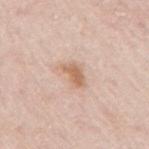Case summary:
- follow-up: no biopsy performed (imaged during a skin exam)
- imaging modality: 15 mm crop, total-body photography
- location: the right upper arm
- subject: female, aged 63–67
- automated metrics: a footprint of about 5 mm², a shape eccentricity near 0.8, and a shape-asymmetry score of about 0.35 (0 = symmetric); a border-irregularity rating of about 3.5/10, internal color variation of about 3 on a 0–10 scale, and radial color variation of about 1; a classifier nevus-likeness of about 85/100 and a lesion-detection confidence of about 100/100
- lighting: white-light